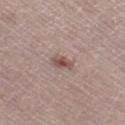notes — no biopsy performed (imaged during a skin exam)
size — ~2.5 mm (longest diameter)
subject — female, roughly 50 years of age
TBP lesion metrics — a shape eccentricity near 0.75 and a shape-asymmetry score of about 0.25 (0 = symmetric); a border-irregularity index near 2/10 and internal color variation of about 4 on a 0–10 scale; an automated nevus-likeness rating near 70 out of 100
lighting — white-light illumination
acquisition — ~15 mm crop, total-body skin-cancer survey
site — the leg